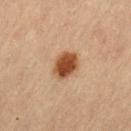Clinical impression: Imaged during a routine full-body skin examination; the lesion was not biopsied and no histopathology is available. Image and clinical context: On the left thigh. A female patient, approximately 65 years of age. A 15 mm close-up extracted from a 3D total-body photography capture. Automated image analysis of the tile measured a lesion color around L≈42 a*≈21 b*≈32 in CIELAB, roughly 14 lightness units darker than nearby skin, and a normalized lesion–skin contrast near 11.5.